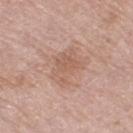The lesion was tiled from a total-body skin photograph and was not biopsied.
A female patient roughly 70 years of age.
The tile uses white-light illumination.
A lesion tile, about 15 mm wide, cut from a 3D total-body photograph.
From the right thigh.
Automated tile analysis of the lesion measured a border-irregularity index near 3.5/10 and a peripheral color-asymmetry measure near 1.